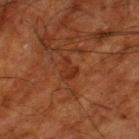Impression:
No biopsy was performed on this lesion — it was imaged during a full skin examination and was not determined to be concerning.
Acquisition and patient details:
Captured under cross-polarized illumination. A roughly 15 mm field-of-view crop from a total-body skin photograph. The lesion is located on the left thigh. An algorithmic analysis of the crop reported a shape eccentricity near 0.7 and two-axis asymmetry of about 0.55. It also reported an average lesion color of about L≈24 a*≈22 b*≈27 (CIELAB) and roughly 5 lightness units darker than nearby skin. Approximately 2.5 mm at its widest. A male patient aged approximately 80.